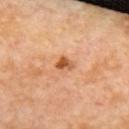Q: Is there a histopathology result?
A: total-body-photography surveillance lesion; no biopsy
Q: What is the lesion's diameter?
A: ≈2.5 mm
Q: What lighting was used for the tile?
A: cross-polarized
Q: What is the anatomic site?
A: the upper back
Q: Who is the patient?
A: female, aged approximately 50
Q: What is the imaging modality?
A: total-body-photography crop, ~15 mm field of view
Q: What did automated image analysis measure?
A: an outline eccentricity of about 0.75 (0 = round, 1 = elongated) and a shape-asymmetry score of about 0.5 (0 = symmetric); a mean CIELAB color near L≈55 a*≈26 b*≈40